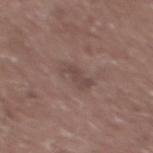Recorded during total-body skin imaging; not selected for excision or biopsy. A 15 mm close-up tile from a total-body photography series done for melanoma screening. Automated tile analysis of the lesion measured a footprint of about 5 mm² and an eccentricity of roughly 0.85. And it measured a mean CIELAB color near L≈45 a*≈16 b*≈20 and a lesion–skin lightness drop of about 7. The software also gave an automated nevus-likeness rating near 0 out of 100 and lesion-presence confidence of about 100/100. The subject is a male about 60 years old. The lesion is on the upper back. This is a white-light tile. The recorded lesion diameter is about 3 mm.